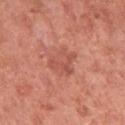| field | value |
|---|---|
| biopsy status | total-body-photography surveillance lesion; no biopsy |
| subject | female, roughly 50 years of age |
| image-analysis metrics | an outline eccentricity of about 0.55 (0 = round, 1 = elongated); a lesion color around L≈54 a*≈29 b*≈31 in CIELAB and a normalized border contrast of about 5; a border-irregularity index near 4/10, a color-variation rating of about 3.5/10, and peripheral color asymmetry of about 1; a lesion-detection confidence of about 100/100 |
| image source | 15 mm crop, total-body photography |
| anatomic site | the left upper arm |